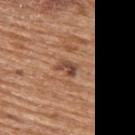Assessment:
No biopsy was performed on this lesion — it was imaged during a full skin examination and was not determined to be concerning.
Clinical summary:
A female subject aged 68 to 72. The lesion-visualizer software estimated an average lesion color of about L≈47 a*≈22 b*≈30 (CIELAB), about 11 CIELAB-L* units darker than the surrounding skin, and a lesion-to-skin contrast of about 8.5 (normalized; higher = more distinct). And it measured a border-irregularity index near 3/10, a within-lesion color-variation index near 3.5/10, and a peripheral color-asymmetry measure near 1.5. Imaged with white-light lighting. Located on the upper back. Longest diameter approximately 2.5 mm. A 15 mm crop from a total-body photograph taken for skin-cancer surveillance.